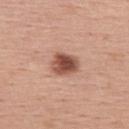Case summary:
• biopsy status: imaged on a skin check; not biopsied
• lighting: white-light
• diameter: ~3.5 mm (longest diameter)
• location: the upper back
• image-analysis metrics: a border-irregularity rating of about 2/10, a color-variation rating of about 6/10, and a peripheral color-asymmetry measure near 2
• patient: male, aged around 55
• image: 15 mm crop, total-body photography